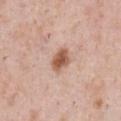Captured during whole-body skin photography for melanoma surveillance; the lesion was not biopsied.